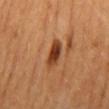follow-up: total-body-photography surveillance lesion; no biopsy
lesion diameter: about 3.5 mm
location: the mid back
image-analysis metrics: roughly 14 lightness units darker than nearby skin and a normalized lesion–skin contrast near 11; a peripheral color-asymmetry measure near 1.5; an automated nevus-likeness rating near 100 out of 100
patient: female
acquisition: 15 mm crop, total-body photography
lighting: cross-polarized illumination